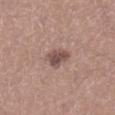biopsy_status: not biopsied; imaged during a skin examination
patient:
  sex: male
  age_approx: 30
image:
  source: total-body photography crop
  field_of_view_mm: 15